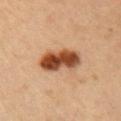Findings:
• notes · no biopsy performed (imaged during a skin exam)
• site · the arm
• imaging modality · 15 mm crop, total-body photography
• illumination · cross-polarized
• automated lesion analysis · a mean CIELAB color near L≈48 a*≈24 b*≈35, roughly 19 lightness units darker than nearby skin, and a lesion-to-skin contrast of about 12.5 (normalized; higher = more distinct); an automated nevus-likeness rating near 100 out of 100
• diameter · ≈5.5 mm
• subject · male, aged 53 to 57